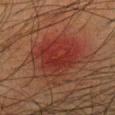  biopsy_status: not biopsied; imaged during a skin examination
  image:
    source: total-body photography crop
    field_of_view_mm: 15
  patient:
    sex: male
    age_approx: 35
  site: left lower leg